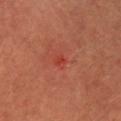{"biopsy_status": "not biopsied; imaged during a skin examination", "site": "head or neck", "patient": {"sex": "male", "age_approx": 50}, "lesion_size": {"long_diameter_mm_approx": 1.5}, "lighting": "cross-polarized", "image": {"source": "total-body photography crop", "field_of_view_mm": 15}}